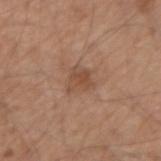Q: Was this lesion biopsied?
A: catalogued during a skin exam; not biopsied
Q: What is the lesion's diameter?
A: ~3 mm (longest diameter)
Q: What is the imaging modality?
A: ~15 mm tile from a whole-body skin photo
Q: What lighting was used for the tile?
A: white-light
Q: Patient demographics?
A: male, aged approximately 55
Q: What is the anatomic site?
A: the right upper arm
Q: Automated lesion metrics?
A: a classifier nevus-likeness of about 10/100 and lesion-presence confidence of about 100/100